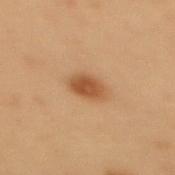– workup — no biopsy performed (imaged during a skin exam)
– lesion size — ≈3.5 mm
– acquisition — 15 mm crop, total-body photography
– lighting — cross-polarized illumination
– body site — the back
– patient — female, about 40 years old